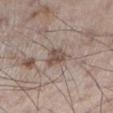Q: Was a biopsy performed?
A: imaged on a skin check; not biopsied
Q: What are the patient's age and sex?
A: male, aged 58 to 62
Q: What is the anatomic site?
A: the left lower leg
Q: How was this image acquired?
A: ~15 mm crop, total-body skin-cancer survey